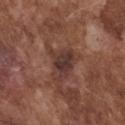Recorded during total-body skin imaging; not selected for excision or biopsy. The patient is a male approximately 75 years of age. A 15 mm close-up tile from a total-body photography series done for melanoma screening. From the chest.